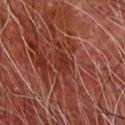biopsy status: total-body-photography surveillance lesion; no biopsy | anatomic site: the arm | image source: total-body-photography crop, ~15 mm field of view | patient: male, approximately 80 years of age.